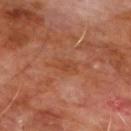{
  "lighting": "cross-polarized",
  "image": {
    "source": "total-body photography crop",
    "field_of_view_mm": 15
  },
  "patient": {
    "sex": "male",
    "age_approx": 60
  },
  "site": "chest"
}